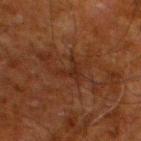Automated image analysis of the tile measured a lesion color around L≈21 a*≈18 b*≈24 in CIELAB and roughly 4 lightness units darker than nearby skin. The software also gave a border-irregularity rating of about 8.5/10, a within-lesion color-variation index near 0.5/10, and a peripheral color-asymmetry measure near 0. The software also gave an automated nevus-likeness rating near 0 out of 100 and a detector confidence of about 80 out of 100 that the crop contains a lesion. The patient is a male aged approximately 80. The lesion is located on the left lower leg. The tile uses cross-polarized illumination. Measured at roughly 3 mm in maximum diameter. A region of skin cropped from a whole-body photographic capture, roughly 15 mm wide.